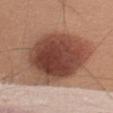Assessment:
No biopsy was performed on this lesion — it was imaged during a full skin examination and was not determined to be concerning.
Context:
Cropped from a total-body skin-imaging series; the visible field is about 15 mm. A male patient, aged 48–52. On the left upper arm. Longest diameter approximately 9.5 mm. Captured under white-light illumination.